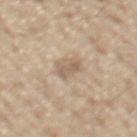Impression:
Imaged during a routine full-body skin examination; the lesion was not biopsied and no histopathology is available.
Context:
A region of skin cropped from a whole-body photographic capture, roughly 15 mm wide. A male subject, about 70 years old. On the mid back.